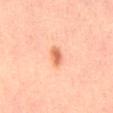Recorded during total-body skin imaging; not selected for excision or biopsy. Automated tile analysis of the lesion measured a lesion area of about 4 mm², a shape eccentricity near 0.85, and a symmetry-axis asymmetry near 0.2. Located on the mid back. Imaged with cross-polarized lighting. Cropped from a whole-body photographic skin survey; the tile spans about 15 mm. A male patient, approximately 30 years of age. The recorded lesion diameter is about 3 mm.